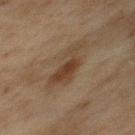No biopsy was performed on this lesion — it was imaged during a full skin examination and was not determined to be concerning.
A 15 mm crop from a total-body photograph taken for skin-cancer surveillance.
The patient is a male approximately 75 years of age.
From the chest.